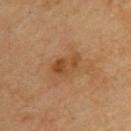{
  "biopsy_status": "not biopsied; imaged during a skin examination",
  "patient": {
    "sex": "male",
    "age_approx": 70
  },
  "lighting": "cross-polarized",
  "lesion_size": {
    "long_diameter_mm_approx": 3.5
  },
  "site": "upper back",
  "automated_metrics": {
    "cielab_L": 44,
    "cielab_a": 20,
    "cielab_b": 36,
    "vs_skin_darker_L": 8.0,
    "vs_skin_contrast_norm": 7.0
  },
  "image": {
    "source": "total-body photography crop",
    "field_of_view_mm": 15
  }
}